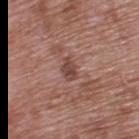| key | value |
|---|---|
| lesion size | ~2.5 mm (longest diameter) |
| acquisition | 15 mm crop, total-body photography |
| anatomic site | the upper back |
| subject | male, aged around 70 |
| tile lighting | white-light |